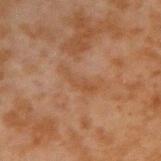The lesion was tiled from a total-body skin photograph and was not biopsied. Captured under cross-polarized illumination. Automated image analysis of the tile measured a footprint of about 5.5 mm² and two-axis asymmetry of about 0.55. It also reported a nevus-likeness score of about 0/100 and a lesion-detection confidence of about 100/100. Longest diameter approximately 4.5 mm. A lesion tile, about 15 mm wide, cut from a 3D total-body photograph. The lesion is on the arm. A male patient, aged around 45.